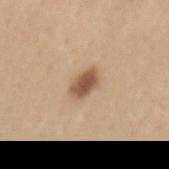biopsy status: no biopsy performed (imaged during a skin exam) | imaging modality: total-body-photography crop, ~15 mm field of view | tile lighting: white-light illumination | patient: female, approximately 35 years of age | anatomic site: the mid back.